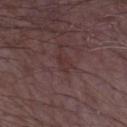{
  "biopsy_status": "not biopsied; imaged during a skin examination",
  "image": {
    "source": "total-body photography crop",
    "field_of_view_mm": 15
  },
  "lesion_size": {
    "long_diameter_mm_approx": 2.5
  },
  "automated_metrics": {
    "area_mm2_approx": 3.0,
    "eccentricity": 0.85,
    "shape_asymmetry": 0.4,
    "cielab_L": 32,
    "cielab_a": 19,
    "cielab_b": 17,
    "vs_skin_darker_L": 4.0,
    "lesion_detection_confidence_0_100": 55
  },
  "lighting": "white-light",
  "patient": {
    "sex": "male",
    "age_approx": 65
  },
  "site": "arm"
}